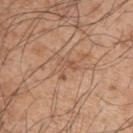<case>
  <biopsy_status>not biopsied; imaged during a skin examination</biopsy_status>
  <patient>
    <sex>male</sex>
    <age_approx>65</age_approx>
  </patient>
  <site>left upper arm</site>
  <image>
    <source>total-body photography crop</source>
    <field_of_view_mm>15</field_of_view_mm>
  </image>
  <lighting>white-light</lighting>
</case>